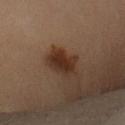The lesion was photographed on a routine skin check and not biopsied; there is no pathology result. On the left upper arm. A roughly 15 mm field-of-view crop from a total-body skin photograph. A female patient in their 60s.